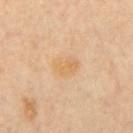The lesion was photographed on a routine skin check and not biopsied; there is no pathology result.
A male subject, approximately 60 years of age.
A region of skin cropped from a whole-body photographic capture, roughly 15 mm wide.
The tile uses cross-polarized illumination.
On the mid back.
The lesion's longest dimension is about 3 mm.